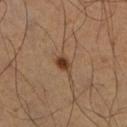Recorded during total-body skin imaging; not selected for excision or biopsy. A male patient, approximately 55 years of age. A lesion tile, about 15 mm wide, cut from a 3D total-body photograph. Measured at roughly 2.5 mm in maximum diameter. The lesion is located on the right lower leg.